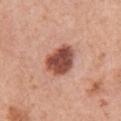Case summary:
– notes — catalogued during a skin exam; not biopsied
– imaging modality — 15 mm crop, total-body photography
– lesion size — ~4 mm (longest diameter)
– location — the right upper arm
– tile lighting — white-light illumination
– patient — female, aged 58 to 62